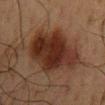Case summary:
– follow-up: catalogued during a skin exam; not biopsied
– patient: male, approximately 60 years of age
– automated lesion analysis: a border-irregularity index near 3/10, internal color variation of about 4.5 on a 0–10 scale, and radial color variation of about 1.5
– image: ~15 mm tile from a whole-body skin photo
– location: the mid back